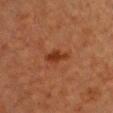{
  "biopsy_status": "not biopsied; imaged during a skin examination",
  "automated_metrics": {
    "area_mm2_approx": 4.0,
    "shape_asymmetry": 0.3,
    "border_irregularity_0_10": 3.0,
    "color_variation_0_10": 1.0,
    "peripheral_color_asymmetry": 0.5
  },
  "lesion_size": {
    "long_diameter_mm_approx": 3.0
  },
  "site": "chest",
  "image": {
    "source": "total-body photography crop",
    "field_of_view_mm": 15
  },
  "lighting": "cross-polarized",
  "patient": {
    "sex": "female",
    "age_approx": 40
  }
}